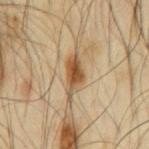biopsy status: catalogued during a skin exam; not biopsied
diameter: ≈5 mm
acquisition: ~15 mm crop, total-body skin-cancer survey
site: the back
patient: male, in their mid- to late 60s
lighting: cross-polarized illumination